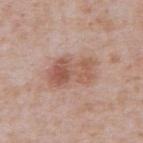Notes:
- notes — catalogued during a skin exam; not biopsied
- image — ~15 mm tile from a whole-body skin photo
- patient — male, aged 63 to 67
- location — the abdomen